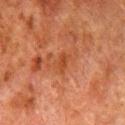Q: Was a biopsy performed?
A: total-body-photography surveillance lesion; no biopsy
Q: What kind of image is this?
A: total-body-photography crop, ~15 mm field of view
Q: Automated lesion metrics?
A: a footprint of about 3.5 mm², an outline eccentricity of about 0.8 (0 = round, 1 = elongated), and a shape-asymmetry score of about 0.4 (0 = symmetric); an average lesion color of about L≈37 a*≈24 b*≈33 (CIELAB) and about 6 CIELAB-L* units darker than the surrounding skin; a border-irregularity rating of about 4/10 and a color-variation rating of about 2/10; a classifier nevus-likeness of about 0/100 and a detector confidence of about 100 out of 100 that the crop contains a lesion
Q: Who is the patient?
A: male, roughly 80 years of age
Q: Where on the body is the lesion?
A: the right lower leg
Q: What lighting was used for the tile?
A: cross-polarized illumination